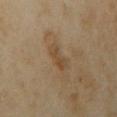Assessment: Part of a total-body skin-imaging series; this lesion was reviewed on a skin check and was not flagged for biopsy. Context: A lesion tile, about 15 mm wide, cut from a 3D total-body photograph. A female patient aged 33 to 37. Located on the right upper arm.